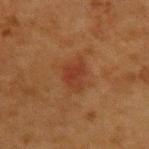Assessment: The lesion was photographed on a routine skin check and not biopsied; there is no pathology result. Background: The lesion is located on the upper back. An algorithmic analysis of the crop reported lesion-presence confidence of about 100/100. The lesion's longest dimension is about 3.5 mm. A female patient, aged around 40. Cropped from a total-body skin-imaging series; the visible field is about 15 mm.